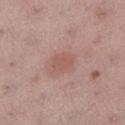Impression: This lesion was catalogued during total-body skin photography and was not selected for biopsy. Clinical summary: This image is a 15 mm lesion crop taken from a total-body photograph. The patient is a female approximately 55 years of age. The lesion is on the left lower leg.